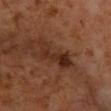Clinical impression:
Imaged during a routine full-body skin examination; the lesion was not biopsied and no histopathology is available.
Background:
Captured under cross-polarized illumination. The lesion is located on the right forearm. The patient is a male aged 58–62. This image is a 15 mm lesion crop taken from a total-body photograph.